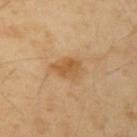Impression: Captured during whole-body skin photography for melanoma surveillance; the lesion was not biopsied. Acquisition and patient details: This is a cross-polarized tile. Longest diameter approximately 3.5 mm. From the left upper arm. A male patient aged 48 to 52. A lesion tile, about 15 mm wide, cut from a 3D total-body photograph.